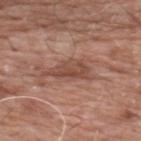location = the upper back; subject = male, roughly 60 years of age; imaging modality = ~15 mm crop, total-body skin-cancer survey.